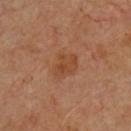Imaged during a routine full-body skin examination; the lesion was not biopsied and no histopathology is available. A male patient, aged approximately 50. The lesion is on the chest. This image is a 15 mm lesion crop taken from a total-body photograph.